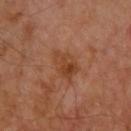Imaged with cross-polarized lighting.
Cropped from a whole-body photographic skin survey; the tile spans about 15 mm.
The lesion is on the upper back.
Automated image analysis of the tile measured an outline eccentricity of about 0.75 (0 = round, 1 = elongated) and a symmetry-axis asymmetry near 0.35. The software also gave a lesion–skin lightness drop of about 7 and a lesion-to-skin contrast of about 6.5 (normalized; higher = more distinct). The software also gave a border-irregularity rating of about 3.5/10, internal color variation of about 4.5 on a 0–10 scale, and radial color variation of about 1.5. It also reported a nevus-likeness score of about 10/100 and a detector confidence of about 100 out of 100 that the crop contains a lesion.
A male patient, aged 53 to 57.